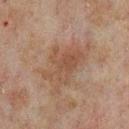From the chest.
A male subject, about 60 years old.
A region of skin cropped from a whole-body photographic capture, roughly 15 mm wide.
This is a cross-polarized tile.